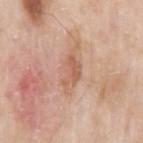Imaged during a routine full-body skin examination; the lesion was not biopsied and no histopathology is available. A male subject aged approximately 55. From the arm. This image is a 15 mm lesion crop taken from a total-body photograph.